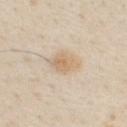<record>
<biopsy_status>not biopsied; imaged during a skin examination</biopsy_status>
<lesion_size>
  <long_diameter_mm_approx>3.5</long_diameter_mm_approx>
</lesion_size>
<automated_metrics>
  <area_mm2_approx>5.0</area_mm2_approx>
  <eccentricity>0.75</eccentricity>
  <shape_asymmetry>0.2</shape_asymmetry>
  <cielab_L>68</cielab_L>
  <cielab_a>14</cielab_a>
  <cielab_b>34</cielab_b>
  <vs_skin_darker_L>8.0</vs_skin_darker_L>
  <vs_skin_contrast_norm>6.5</vs_skin_contrast_norm>
  <border_irregularity_0_10>2.0</border_irregularity_0_10>
  <color_variation_0_10>3.0</color_variation_0_10>
  <peripheral_color_asymmetry>1.0</peripheral_color_asymmetry>
  <nevus_likeness_0_100>70</nevus_likeness_0_100>
  <lesion_detection_confidence_0_100>100</lesion_detection_confidence_0_100>
</automated_metrics>
<site>chest</site>
<patient>
  <sex>male</sex>
  <age_approx>30</age_approx>
</patient>
<image>
  <source>total-body photography crop</source>
  <field_of_view_mm>15</field_of_view_mm>
</image>
</record>